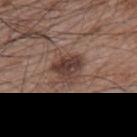No biopsy was performed on this lesion — it was imaged during a full skin examination and was not determined to be concerning.
From the upper back.
Imaged with white-light lighting.
A male subject, in their mid-60s.
Cropped from a total-body skin-imaging series; the visible field is about 15 mm.
The recorded lesion diameter is about 3.5 mm.